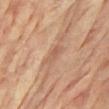notes = imaged on a skin check; not biopsied | TBP lesion metrics = a lesion color around L≈51 a*≈19 b*≈28 in CIELAB, roughly 7 lightness units darker than nearby skin, and a normalized border contrast of about 5; a within-lesion color-variation index near 1/10 | site = the right arm | patient = female, aged around 80 | illumination = cross-polarized illumination | lesion size = ≈2.5 mm | acquisition = 15 mm crop, total-body photography.